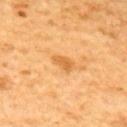Findings:
• biopsy status: no biopsy performed (imaged during a skin exam)
• location: the upper back
• image source: ~15 mm crop, total-body skin-cancer survey
• subject: male, about 60 years old
• diameter: ≈3 mm
• image-analysis metrics: a footprint of about 3.5 mm², an outline eccentricity of about 0.85 (0 = round, 1 = elongated), and a shape-asymmetry score of about 0.25 (0 = symmetric); a lesion color around L≈63 a*≈26 b*≈49 in CIELAB, roughly 10 lightness units darker than nearby skin, and a normalized border contrast of about 6.5